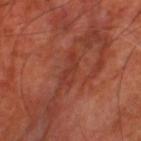Impression: Recorded during total-body skin imaging; not selected for excision or biopsy. Background: Captured under cross-polarized illumination. A lesion tile, about 15 mm wide, cut from a 3D total-body photograph. About 3.5 mm across. A subject approximately 65 years of age. The lesion is located on the left thigh.